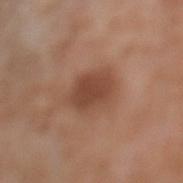This lesion was catalogued during total-body skin photography and was not selected for biopsy. The lesion is located on the left lower leg. A 15 mm crop from a total-body photograph taken for skin-cancer surveillance. Imaged with white-light lighting. Automated tile analysis of the lesion measured an area of roughly 13 mm², an eccentricity of roughly 0.65, and a shape-asymmetry score of about 0.15 (0 = symmetric). The software also gave a border-irregularity index near 2/10. About 5 mm across. A male subject, approximately 80 years of age.